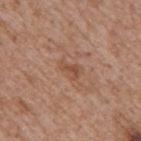Assessment:
Recorded during total-body skin imaging; not selected for excision or biopsy.
Context:
A close-up tile cropped from a whole-body skin photograph, about 15 mm across. Imaged with white-light lighting. The lesion is located on the back. A male subject, aged around 65. The lesion-visualizer software estimated a lesion color around L≈50 a*≈21 b*≈31 in CIELAB, a lesion–skin lightness drop of about 7, and a lesion-to-skin contrast of about 5.5 (normalized; higher = more distinct). It also reported a classifier nevus-likeness of about 0/100 and a detector confidence of about 100 out of 100 that the crop contains a lesion.